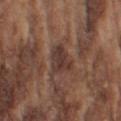Q: Was this lesion biopsied?
A: total-body-photography surveillance lesion; no biopsy
Q: What is the anatomic site?
A: the left upper arm
Q: What did automated image analysis measure?
A: about 9 CIELAB-L* units darker than the surrounding skin
Q: How was the tile lit?
A: white-light illumination
Q: What are the patient's age and sex?
A: male, aged around 75
Q: What kind of image is this?
A: ~15 mm tile from a whole-body skin photo
Q: Lesion size?
A: ≈3 mm